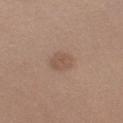Clinical impression:
Imaged during a routine full-body skin examination; the lesion was not biopsied and no histopathology is available.
Context:
The lesion's longest dimension is about 3 mm. A lesion tile, about 15 mm wide, cut from a 3D total-body photograph. A male patient, aged 28–32. From the left upper arm. Captured under white-light illumination.